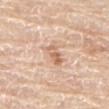| feature | finding |
|---|---|
| workup | no biopsy performed (imaged during a skin exam) |
| subject | male, roughly 80 years of age |
| diameter | ≈3 mm |
| anatomic site | the abdomen |
| automated lesion analysis | a mean CIELAB color near L≈65 a*≈20 b*≈32, roughly 10 lightness units darker than nearby skin, and a normalized border contrast of about 6.5 |
| illumination | white-light illumination |
| imaging modality | ~15 mm tile from a whole-body skin photo |